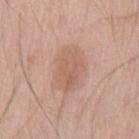Notes:
• biopsy status — total-body-photography surveillance lesion; no biopsy
• lesion size — about 4.5 mm
• location — the mid back
• acquisition — ~15 mm tile from a whole-body skin photo
• illumination — white-light illumination
• TBP lesion metrics — a footprint of about 13 mm², an eccentricity of roughly 0.7, and two-axis asymmetry of about 0.15; an automated nevus-likeness rating near 20 out of 100 and a detector confidence of about 100 out of 100 that the crop contains a lesion
• patient — male, approximately 70 years of age